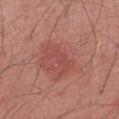  biopsy_status: not biopsied; imaged during a skin examination
  image:
    source: total-body photography crop
    field_of_view_mm: 15
  lesion_size:
    long_diameter_mm_approx: 4.0
  lighting: white-light
  site: left forearm
  patient:
    sex: male
    age_approx: 50
  automated_metrics:
    border_irregularity_0_10: 6.5
    nevus_likeness_0_100: 0
    lesion_detection_confidence_0_100: 100